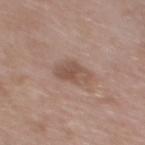Acquisition and patient details:
This is a white-light tile. A female subject, aged 48 to 52. An algorithmic analysis of the crop reported a lesion area of about 7 mm² and a symmetry-axis asymmetry near 0.25. It also reported a mean CIELAB color near L≈52 a*≈18 b*≈26, a lesion–skin lightness drop of about 9, and a normalized border contrast of about 6.5. Located on the upper back. A 15 mm crop from a total-body photograph taken for skin-cancer surveillance. Longest diameter approximately 4 mm.